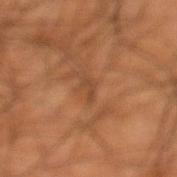biopsy status = catalogued during a skin exam; not biopsied
image = 15 mm crop, total-body photography
body site = the left lower leg
patient = male, about 55 years old
lesion diameter = ~2.5 mm (longest diameter)
automated metrics = an area of roughly 2 mm² and a shape eccentricity near 0.95; an average lesion color of about L≈40 a*≈20 b*≈31 (CIELAB), a lesion–skin lightness drop of about 7, and a normalized lesion–skin contrast near 5.5; a nevus-likeness score of about 0/100 and lesion-presence confidence of about 75/100
illumination = cross-polarized